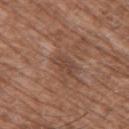The lesion was photographed on a routine skin check and not biopsied; there is no pathology result. The patient is a male approximately 60 years of age. This image is a 15 mm lesion crop taken from a total-body photograph. Longest diameter approximately 3 mm. The tile uses white-light illumination. Automated tile analysis of the lesion measured a lesion–skin lightness drop of about 7 and a normalized border contrast of about 5.5. The analysis additionally found a border-irregularity index near 4.5/10, internal color variation of about 1 on a 0–10 scale, and peripheral color asymmetry of about 0.5. And it measured a lesion-detection confidence of about 90/100. On the chest.